The total-body-photography lesion software estimated an area of roughly 50 mm², an eccentricity of roughly 0.8, and two-axis asymmetry of about 0.1. And it measured a border-irregularity index near 1.5/10, a within-lesion color-variation index near 7.5/10, and a peripheral color-asymmetry measure near 2. The analysis additionally found lesion-presence confidence of about 100/100; the subject is a male aged 43 to 47; a roughly 15 mm field-of-view crop from a total-body skin photograph; imaged with white-light lighting; the lesion's longest dimension is about 10.5 mm; the lesion is located on the back.
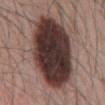Diagnosis:
On excision, pathology confirmed an atypical intraepithelial melanocytic proliferation, classified as an indeterminate (borderline) lesion.